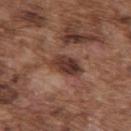The lesion was photographed on a routine skin check and not biopsied; there is no pathology result. On the upper back. A male subject, aged 73 to 77. Cropped from a total-body skin-imaging series; the visible field is about 15 mm. This is a white-light tile. Automated image analysis of the tile measured a shape eccentricity near 0.85 and two-axis asymmetry of about 0.3. The software also gave an average lesion color of about L≈37 a*≈21 b*≈25 (CIELAB) and a lesion–skin lightness drop of about 12. It also reported border irregularity of about 3 on a 0–10 scale, a within-lesion color-variation index near 5.5/10, and peripheral color asymmetry of about 2.